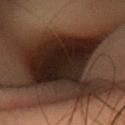{
  "biopsy_status": "not biopsied; imaged during a skin examination",
  "patient": {
    "sex": "female",
    "age_approx": 40
  },
  "image": {
    "source": "total-body photography crop",
    "field_of_view_mm": 15
  },
  "site": "head or neck"
}